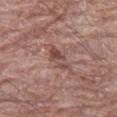<tbp_lesion>
  <automated_metrics>
    <area_mm2_approx>5.0</area_mm2_approx>
    <eccentricity>0.9</eccentricity>
    <shape_asymmetry>0.3</shape_asymmetry>
    <border_irregularity_0_10>3.5</border_irregularity_0_10>
    <color_variation_0_10>7.0</color_variation_0_10>
    <peripheral_color_asymmetry>3.0</peripheral_color_asymmetry>
    <nevus_likeness_0_100>0</nevus_likeness_0_100>
  </automated_metrics>
  <image>
    <source>total-body photography crop</source>
    <field_of_view_mm>15</field_of_view_mm>
  </image>
  <patient>
    <sex>male</sex>
    <age_approx>70</age_approx>
  </patient>
  <site>leg</site>
  <lesion_size>
    <long_diameter_mm_approx>4.0</long_diameter_mm_approx>
  </lesion_size>
</tbp_lesion>